The lesion is on the arm; the recorded lesion diameter is about 10 mm; a male subject, roughly 55 years of age; the tile uses white-light illumination; cropped from a total-body skin-imaging series; the visible field is about 15 mm.
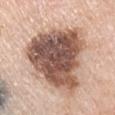Conclusion:
On excision, pathology confirmed an atypical intraepithelial melanocytic proliferation (borderline).